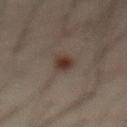biopsy_status: not biopsied; imaged during a skin examination
patient:
  sex: male
  age_approx: 50
lesion_size:
  long_diameter_mm_approx: 3.0
lighting: cross-polarized
site: abdomen
image:
  source: total-body photography crop
  field_of_view_mm: 15
automated_metrics:
  area_mm2_approx: 4.0